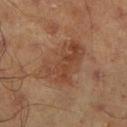follow-up = imaged on a skin check; not biopsied | tile lighting = cross-polarized | lesion size = ≈6 mm | anatomic site = the right lower leg | subject = male, aged 43–47 | imaging modality = ~15 mm tile from a whole-body skin photo.